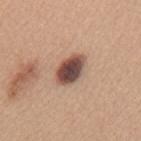follow-up = catalogued during a skin exam; not biopsied
anatomic site = the upper back
image = ~15 mm crop, total-body skin-cancer survey
lesion size = about 4 mm
tile lighting = white-light illumination
subject = female, aged 38 to 42